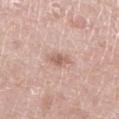Captured during whole-body skin photography for melanoma surveillance; the lesion was not biopsied.
The tile uses white-light illumination.
The lesion-visualizer software estimated radial color variation of about 0.5.
A 15 mm crop from a total-body photograph taken for skin-cancer surveillance.
Longest diameter approximately 3 mm.
From the left lower leg.
A female patient in their 70s.